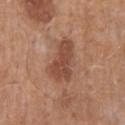Part of a total-body skin-imaging series; this lesion was reviewed on a skin check and was not flagged for biopsy. The subject is a male aged around 70. This is a white-light tile. A 15 mm close-up tile from a total-body photography series done for melanoma screening. Longest diameter approximately 5.5 mm. The lesion is on the mid back.